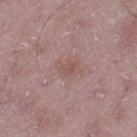The lesion is located on the left thigh.
A male subject, aged 48–52.
This image is a 15 mm lesion crop taken from a total-body photograph.